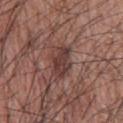Clinical impression:
Imaged during a routine full-body skin examination; the lesion was not biopsied and no histopathology is available.
Acquisition and patient details:
Located on the upper back. A male subject, aged approximately 70. Measured at roughly 4 mm in maximum diameter. Cropped from a total-body skin-imaging series; the visible field is about 15 mm. Automated tile analysis of the lesion measured a footprint of about 7.5 mm² and a shape eccentricity near 0.8. It also reported a lesion color around L≈38 a*≈19 b*≈21 in CIELAB, about 10 CIELAB-L* units darker than the surrounding skin, and a lesion-to-skin contrast of about 8 (normalized; higher = more distinct). And it measured border irregularity of about 3 on a 0–10 scale and a within-lesion color-variation index near 3/10. It also reported a nevus-likeness score of about 15/100 and a lesion-detection confidence of about 95/100.